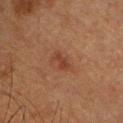| field | value |
|---|---|
| biopsy status | total-body-photography surveillance lesion; no biopsy |
| illumination | cross-polarized illumination |
| size | about 2.5 mm |
| anatomic site | the front of the torso |
| image source | ~15 mm crop, total-body skin-cancer survey |
| subject | male, approximately 50 years of age |
| image-analysis metrics | an area of roughly 3 mm² and a shape-asymmetry score of about 0.3 (0 = symmetric); internal color variation of about 0.5 on a 0–10 scale and radial color variation of about 0; a classifier nevus-likeness of about 10/100 and a lesion-detection confidence of about 100/100 |